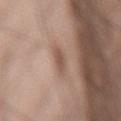Clinical impression:
The lesion was photographed on a routine skin check and not biopsied; there is no pathology result.